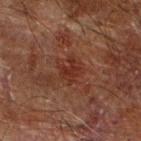Notes:
* lighting: cross-polarized
* body site: the right forearm
* patient: male, in their mid- to late 60s
* size: ≈2.5 mm
* imaging modality: 15 mm crop, total-body photography
* automated lesion analysis: about 6 CIELAB-L* units darker than the surrounding skin and a lesion-to-skin contrast of about 7 (normalized; higher = more distinct); border irregularity of about 4 on a 0–10 scale, a color-variation rating of about 2/10, and a peripheral color-asymmetry measure near 1; an automated nevus-likeness rating near 5 out of 100